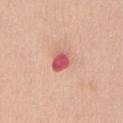This lesion was catalogued during total-body skin photography and was not selected for biopsy. From the chest. A roughly 15 mm field-of-view crop from a total-body skin photograph. A male subject in their mid-30s.